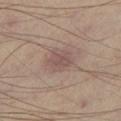workup: no biopsy performed (imaged during a skin exam)
size: ≈3.5 mm
site: the left lower leg
image-analysis metrics: a lesion area of about 7 mm² and a symmetry-axis asymmetry near 0.25; a mean CIELAB color near L≈52 a*≈17 b*≈21, about 8 CIELAB-L* units darker than the surrounding skin, and a lesion-to-skin contrast of about 6 (normalized; higher = more distinct)
image source: 15 mm crop, total-body photography
subject: male, aged approximately 65